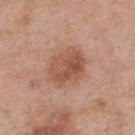This lesion was catalogued during total-body skin photography and was not selected for biopsy.
From the upper back.
A male subject, about 55 years old.
A 15 mm crop from a total-body photograph taken for skin-cancer surveillance.